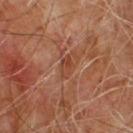This lesion was catalogued during total-body skin photography and was not selected for biopsy.
A region of skin cropped from a whole-body photographic capture, roughly 15 mm wide.
A male patient, about 65 years old.
Measured at roughly 3 mm in maximum diameter.
This is a cross-polarized tile.